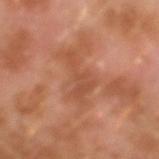<lesion>
  <biopsy_status>not biopsied; imaged during a skin examination</biopsy_status>
  <patient>
    <sex>male</sex>
    <age_approx>30</age_approx>
  </patient>
  <lighting>cross-polarized</lighting>
  <image>
    <source>total-body photography crop</source>
    <field_of_view_mm>15</field_of_view_mm>
  </image>
  <lesion_size>
    <long_diameter_mm_approx>5.0</long_diameter_mm_approx>
  </lesion_size>
  <site>left forearm</site>
  <automated_metrics>
    <area_mm2_approx>8.0</area_mm2_approx>
    <eccentricity>0.85</eccentricity>
    <shape_asymmetry>0.7</shape_asymmetry>
    <border_irregularity_0_10>9.0</border_irregularity_0_10>
    <color_variation_0_10>1.5</color_variation_0_10>
    <peripheral_color_asymmetry>0.5</peripheral_color_asymmetry>
  </automated_metrics>
</lesion>